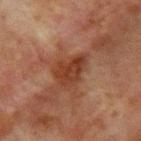Clinical impression: The lesion was photographed on a routine skin check and not biopsied; there is no pathology result. Clinical summary: The tile uses cross-polarized illumination. The patient is a male aged 63–67. A region of skin cropped from a whole-body photographic capture, roughly 15 mm wide. The lesion-visualizer software estimated an area of roughly 9 mm², an outline eccentricity of about 0.7 (0 = round, 1 = elongated), and a symmetry-axis asymmetry near 0.35. The analysis additionally found a mean CIELAB color near L≈31 a*≈21 b*≈27 and about 8 CIELAB-L* units darker than the surrounding skin. It also reported border irregularity of about 4 on a 0–10 scale, internal color variation of about 3 on a 0–10 scale, and a peripheral color-asymmetry measure near 1. And it measured an automated nevus-likeness rating near 0 out of 100. From the chest. The lesion's longest dimension is about 4 mm.